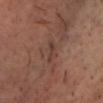patient: male, aged 53–57 | image: ~15 mm crop, total-body skin-cancer survey | image-analysis metrics: a lesion color around L≈37 a*≈19 b*≈22 in CIELAB, about 6 CIELAB-L* units darker than the surrounding skin, and a lesion-to-skin contrast of about 5.5 (normalized; higher = more distinct); internal color variation of about 0 on a 0–10 scale and radial color variation of about 0 | anatomic site: the chest | illumination: cross-polarized illumination.